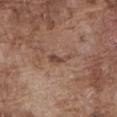– notes — total-body-photography surveillance lesion; no biopsy
– patient — male, about 75 years old
– anatomic site — the abdomen
– image source — ~15 mm crop, total-body skin-cancer survey
– lesion size — ~3 mm (longest diameter)
– tile lighting — white-light